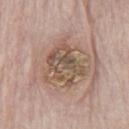Q: Is there a histopathology result?
A: catalogued during a skin exam; not biopsied
Q: What kind of image is this?
A: ~15 mm tile from a whole-body skin photo
Q: Lesion size?
A: ≈5 mm
Q: What are the patient's age and sex?
A: male, approximately 85 years of age or older
Q: Where on the body is the lesion?
A: the chest